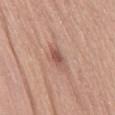size = about 2.5 mm | image = 15 mm crop, total-body photography | location = the back | patient = male, in their mid- to late 40s.